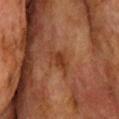The lesion was tiled from a total-body skin photograph and was not biopsied. The total-body-photography lesion software estimated an eccentricity of roughly 0.75 and a shape-asymmetry score of about 0.35 (0 = symmetric). It also reported a border-irregularity rating of about 3.5/10 and a color-variation rating of about 1/10. About 2.5 mm across. Cropped from a total-body skin-imaging series; the visible field is about 15 mm. The tile uses cross-polarized illumination. On the upper back. A male subject, in their mid- to late 70s.